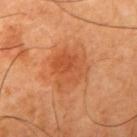Assessment: No biopsy was performed on this lesion — it was imaged during a full skin examination and was not determined to be concerning. Context: Located on the right upper arm. A male subject, aged 63–67. A close-up tile cropped from a whole-body skin photograph, about 15 mm across. Captured under cross-polarized illumination. The lesion's longest dimension is about 5 mm.